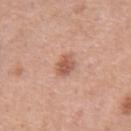Assessment:
Captured during whole-body skin photography for melanoma surveillance; the lesion was not biopsied.
Background:
On the back. A male subject roughly 70 years of age. The total-body-photography lesion software estimated a nevus-likeness score of about 85/100. This is a white-light tile. The recorded lesion diameter is about 2.5 mm. This image is a 15 mm lesion crop taken from a total-body photograph.